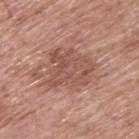follow-up: total-body-photography surveillance lesion; no biopsy | body site: the upper back | automated lesion analysis: a mean CIELAB color near L≈52 a*≈22 b*≈27, roughly 9 lightness units darker than nearby skin, and a lesion-to-skin contrast of about 6 (normalized; higher = more distinct); a nevus-likeness score of about 10/100 and a lesion-detection confidence of about 100/100 | image: ~15 mm crop, total-body skin-cancer survey | tile lighting: white-light | subject: male, about 60 years old.